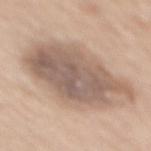Assessment: Imaged during a routine full-body skin examination; the lesion was not biopsied and no histopathology is available. Background: Longest diameter approximately 9.5 mm. The lesion is on the mid back. A female patient aged approximately 65. Captured under white-light illumination. Cropped from a total-body skin-imaging series; the visible field is about 15 mm.